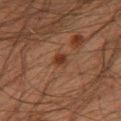Clinical impression:
The lesion was photographed on a routine skin check and not biopsied; there is no pathology result.
Context:
The recorded lesion diameter is about 2 mm. A male subject aged around 35. The lesion is on the right thigh. A close-up tile cropped from a whole-body skin photograph, about 15 mm across. The tile uses cross-polarized illumination.